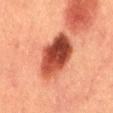  biopsy_status: not biopsied; imaged during a skin examination
  image:
    source: total-body photography crop
    field_of_view_mm: 15
  site: mid back
  patient:
    sex: male
    age_approx: 40
  lesion_size:
    long_diameter_mm_approx: 6.5
  automated_metrics:
    border_irregularity_0_10: 2.0
    peripheral_color_asymmetry: 3.5
  lighting: cross-polarized